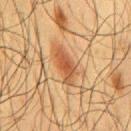The lesion was tiled from a total-body skin photograph and was not biopsied. Located on the chest. The lesion's longest dimension is about 5 mm. A male patient, about 60 years old. A region of skin cropped from a whole-body photographic capture, roughly 15 mm wide.